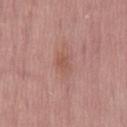Assessment:
The lesion was tiled from a total-body skin photograph and was not biopsied.
Background:
The lesion is located on the back. A male patient, in their mid- to late 50s. A 15 mm crop from a total-body photograph taken for skin-cancer surveillance. Automated image analysis of the tile measured a footprint of about 4 mm², an outline eccentricity of about 0.7 (0 = round, 1 = elongated), and two-axis asymmetry of about 0.25. The software also gave an average lesion color of about L≈54 a*≈22 b*≈27 (CIELAB), about 6 CIELAB-L* units darker than the surrounding skin, and a normalized lesion–skin contrast near 5.5. The software also gave border irregularity of about 2.5 on a 0–10 scale, internal color variation of about 2.5 on a 0–10 scale, and radial color variation of about 1. And it measured a classifier nevus-likeness of about 0/100 and lesion-presence confidence of about 100/100. The lesion's longest dimension is about 2.5 mm. The tile uses white-light illumination.